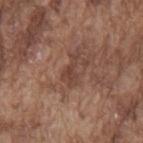Imaged during a routine full-body skin examination; the lesion was not biopsied and no histopathology is available. From the mid back. A male subject approximately 75 years of age. Cropped from a total-body skin-imaging series; the visible field is about 15 mm. Imaged with white-light lighting.